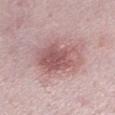The lesion was photographed on a routine skin check and not biopsied; there is no pathology result.
Automated tile analysis of the lesion measured an average lesion color of about L≈56 a*≈23 b*≈20 (CIELAB), roughly 12 lightness units darker than nearby skin, and a lesion-to-skin contrast of about 7.5 (normalized; higher = more distinct). The software also gave a color-variation rating of about 5.5/10 and peripheral color asymmetry of about 2.
A lesion tile, about 15 mm wide, cut from a 3D total-body photograph.
Longest diameter approximately 6 mm.
The patient is a female aged approximately 45.
The lesion is located on the left lower leg.